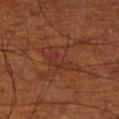  biopsy_status: not biopsied; imaged during a skin examination
  patient:
    sex: male
    age_approx: 70
  site: right lower leg
  lesion_size:
    long_diameter_mm_approx: 4.5
  lighting: cross-polarized
  image:
    source: total-body photography crop
    field_of_view_mm: 15